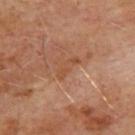Q: Is there a histopathology result?
A: no biopsy performed (imaged during a skin exam)
Q: Who is the patient?
A: male, aged approximately 65
Q: What kind of image is this?
A: ~15 mm crop, total-body skin-cancer survey
Q: Automated lesion metrics?
A: a lesion area of about 3 mm², an outline eccentricity of about 0.95 (0 = round, 1 = elongated), and a symmetry-axis asymmetry near 0.45
Q: Illumination type?
A: cross-polarized illumination
Q: Where on the body is the lesion?
A: the back
Q: How large is the lesion?
A: ≈3 mm